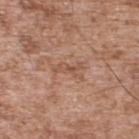Case summary:
– workup · catalogued during a skin exam; not biopsied
– body site · the upper back
– patient · male, aged 53–57
– image source · 15 mm crop, total-body photography
– diameter · about 3.5 mm